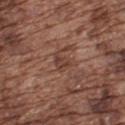automated lesion analysis — a footprint of about 4 mm² and a symmetry-axis asymmetry near 0.45; a lesion color around L≈41 a*≈20 b*≈26 in CIELAB, about 7 CIELAB-L* units darker than the surrounding skin, and a normalized lesion–skin contrast near 6
lighting — white-light illumination
size — ≈2.5 mm
anatomic site — the upper back
image source — 15 mm crop, total-body photography
patient — male, in their mid- to late 70s